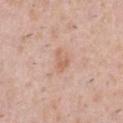Part of a total-body skin-imaging series; this lesion was reviewed on a skin check and was not flagged for biopsy.
A roughly 15 mm field-of-view crop from a total-body skin photograph.
A male subject, about 40 years old.
From the abdomen.